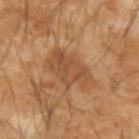Clinical impression:
Imaged during a routine full-body skin examination; the lesion was not biopsied and no histopathology is available.
Background:
Longest diameter approximately 6 mm. A male patient in their mid-50s. On the left forearm. A roughly 15 mm field-of-view crop from a total-body skin photograph.